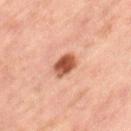| key | value |
|---|---|
| biopsy status | no biopsy performed (imaged during a skin exam) |
| image | total-body-photography crop, ~15 mm field of view |
| diameter | ~3 mm (longest diameter) |
| body site | the left thigh |
| image-analysis metrics | a lesion area of about 6 mm² and two-axis asymmetry of about 0.2; a lesion color around L≈48 a*≈25 b*≈31 in CIELAB, about 15 CIELAB-L* units darker than the surrounding skin, and a normalized border contrast of about 11; a border-irregularity rating of about 2/10; a nevus-likeness score of about 100/100 |
| subject | female, aged 58–62 |
| illumination | cross-polarized illumination |